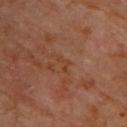Imaged during a routine full-body skin examination; the lesion was not biopsied and no histopathology is available.
Approximately 2.5 mm at its widest.
A male subject, roughly 60 years of age.
The lesion is on the upper back.
A lesion tile, about 15 mm wide, cut from a 3D total-body photograph.
Captured under cross-polarized illumination.